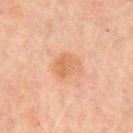Imaged during a routine full-body skin examination; the lesion was not biopsied and no histopathology is available. The lesion-visualizer software estimated a mean CIELAB color near L≈49 a*≈18 b*≈29, a lesion–skin lightness drop of about 6, and a lesion-to-skin contrast of about 5.5 (normalized; higher = more distinct). A 15 mm close-up tile from a total-body photography series done for melanoma screening. The lesion's longest dimension is about 3.5 mm. This is a cross-polarized tile. Located on the chest. A male patient, roughly 60 years of age.